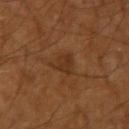Impression: Imaged during a routine full-body skin examination; the lesion was not biopsied and no histopathology is available. Clinical summary: Measured at roughly 2.5 mm in maximum diameter. A male subject aged around 60. The tile uses cross-polarized illumination. A lesion tile, about 15 mm wide, cut from a 3D total-body photograph. Automated tile analysis of the lesion measured a footprint of about 5 mm² and an outline eccentricity of about 0.7 (0 = round, 1 = elongated). The analysis additionally found an automated nevus-likeness rating near 0 out of 100 and a detector confidence of about 100 out of 100 that the crop contains a lesion. The lesion is located on the right upper arm.